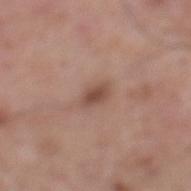The patient is a male roughly 60 years of age. A lesion tile, about 15 mm wide, cut from a 3D total-body photograph. From the mid back.